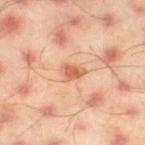The lesion was tiled from a total-body skin photograph and was not biopsied.
Measured at roughly 2.5 mm in maximum diameter.
The lesion is on the right thigh.
An algorithmic analysis of the crop reported a footprint of about 4 mm² and a shape eccentricity near 0.7.
A 15 mm crop from a total-body photograph taken for skin-cancer surveillance.
This is a cross-polarized tile.
A male subject about 45 years old.